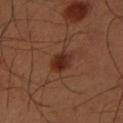Assessment:
Recorded during total-body skin imaging; not selected for excision or biopsy.
Context:
On the left lower leg. Approximately 3.5 mm at its widest. A 15 mm close-up extracted from a 3D total-body photography capture. A male subject, in their 50s. This is a cross-polarized tile.